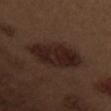No biopsy was performed on this lesion — it was imaged during a full skin examination and was not determined to be concerning. The patient is a male roughly 70 years of age. The lesion is on the left upper arm. This is a white-light tile. A 15 mm close-up tile from a total-body photography series done for melanoma screening. Longest diameter approximately 7 mm.